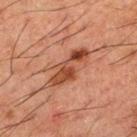<tbp_lesion>
<biopsy_status>not biopsied; imaged during a skin examination</biopsy_status>
<patient>
  <sex>male</sex>
  <age_approx>50</age_approx>
</patient>
<site>upper back</site>
<image>
  <source>total-body photography crop</source>
  <field_of_view_mm>15</field_of_view_mm>
</image>
</tbp_lesion>